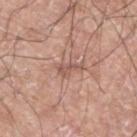Impression:
The lesion was tiled from a total-body skin photograph and was not biopsied.
Context:
From the left lower leg. The subject is a male about 60 years old. A lesion tile, about 15 mm wide, cut from a 3D total-body photograph.